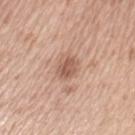biopsy status = total-body-photography surveillance lesion; no biopsy
imaging modality = 15 mm crop, total-body photography
patient = female, about 50 years old
site = the left upper arm